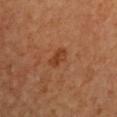Assessment: No biopsy was performed on this lesion — it was imaged during a full skin examination and was not determined to be concerning. Image and clinical context: Imaged with cross-polarized lighting. Automated tile analysis of the lesion measured a shape eccentricity near 0.85. The analysis additionally found a lesion color around L≈39 a*≈24 b*≈34 in CIELAB, roughly 8 lightness units darker than nearby skin, and a normalized lesion–skin contrast near 7.5. The analysis additionally found border irregularity of about 3 on a 0–10 scale, internal color variation of about 1.5 on a 0–10 scale, and peripheral color asymmetry of about 0.5. A 15 mm close-up tile from a total-body photography series done for melanoma screening. From the chest. Approximately 3 mm at its widest. The patient is a male in their 70s.